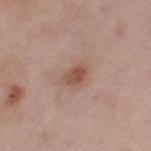{"site": "left thigh", "lighting": "white-light", "patient": {"sex": "female", "age_approx": 65}, "image": {"source": "total-body photography crop", "field_of_view_mm": 15}, "lesion_size": {"long_diameter_mm_approx": 3.0}, "automated_metrics": {"area_mm2_approx": 5.0, "eccentricity": 0.7, "shape_asymmetry": 0.25, "border_irregularity_0_10": 2.0, "peripheral_color_asymmetry": 1.0, "nevus_likeness_0_100": 70, "lesion_detection_confidence_0_100": 100}}